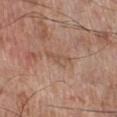follow-up — total-body-photography surveillance lesion; no biopsy | imaging modality — total-body-photography crop, ~15 mm field of view | subject — male, aged approximately 70 | body site — the right lower leg | lighting — white-light illumination | automated lesion analysis — an area of roughly 4 mm², an outline eccentricity of about 0.9 (0 = round, 1 = elongated), and a shape-asymmetry score of about 0.45 (0 = symmetric); a border-irregularity rating of about 5/10, a within-lesion color-variation index near 1.5/10, and radial color variation of about 0.5; a classifier nevus-likeness of about 0/100 and lesion-presence confidence of about 95/100 | lesion diameter — about 3.5 mm.